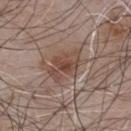Assessment:
Captured during whole-body skin photography for melanoma surveillance; the lesion was not biopsied.
Acquisition and patient details:
The tile uses white-light illumination. The patient is a male roughly 55 years of age. The total-body-photography lesion software estimated a lesion-detection confidence of about 100/100. On the chest. A lesion tile, about 15 mm wide, cut from a 3D total-body photograph.